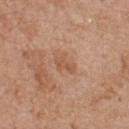{"patient": {"sex": "male", "age_approx": 80}, "lighting": "white-light", "image": {"source": "total-body photography crop", "field_of_view_mm": 15}, "site": "upper back", "automated_metrics": {"area_mm2_approx": 3.5, "shape_asymmetry": 0.45, "cielab_L": 55, "cielab_a": 22, "cielab_b": 33, "vs_skin_contrast_norm": 5.5, "nevus_likeness_0_100": 0, "lesion_detection_confidence_0_100": 100}, "lesion_size": {"long_diameter_mm_approx": 3.0}}